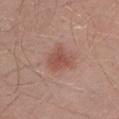Q: What is the lesion's diameter?
A: ≈3.5 mm
Q: Automated lesion metrics?
A: a mean CIELAB color near L≈51 a*≈24 b*≈26, roughly 9 lightness units darker than nearby skin, and a normalized lesion–skin contrast near 6.5
Q: What is the anatomic site?
A: the left lower leg
Q: Patient demographics?
A: male, about 30 years old
Q: What kind of image is this?
A: 15 mm crop, total-body photography